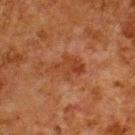* biopsy status: catalogued during a skin exam; not biopsied
* lesion diameter: ~4.5 mm (longest diameter)
* image source: ~15 mm crop, total-body skin-cancer survey
* automated metrics: a mean CIELAB color near L≈33 a*≈22 b*≈30, a lesion–skin lightness drop of about 6, and a normalized lesion–skin contrast near 6; a border-irregularity rating of about 5/10, internal color variation of about 3 on a 0–10 scale, and peripheral color asymmetry of about 1; a classifier nevus-likeness of about 0/100 and lesion-presence confidence of about 100/100
* anatomic site: the upper back
* subject: male, aged 78 to 82
* lighting: cross-polarized illumination